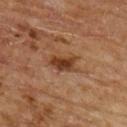About 3.5 mm across.
A close-up tile cropped from a whole-body skin photograph, about 15 mm across.
Captured under cross-polarized illumination.
The total-body-photography lesion software estimated a lesion area of about 6 mm² and an eccentricity of roughly 0.8. It also reported border irregularity of about 3 on a 0–10 scale, a color-variation rating of about 3.5/10, and a peripheral color-asymmetry measure near 1. It also reported an automated nevus-likeness rating near 85 out of 100 and lesion-presence confidence of about 100/100.
The patient is a male in their mid-60s.
On the upper back.